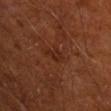Impression:
Captured during whole-body skin photography for melanoma surveillance; the lesion was not biopsied.
Background:
Cropped from a whole-body photographic skin survey; the tile spans about 15 mm. The tile uses cross-polarized illumination. Approximately 2.5 mm at its widest. The lesion is located on the right upper arm. A male subject, in their mid- to late 60s. The lesion-visualizer software estimated a lesion area of about 3.5 mm². The software also gave peripheral color asymmetry of about 1. The analysis additionally found a classifier nevus-likeness of about 0/100 and a detector confidence of about 100 out of 100 that the crop contains a lesion.